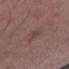Captured during whole-body skin photography for melanoma surveillance; the lesion was not biopsied.